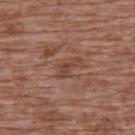This lesion was catalogued during total-body skin photography and was not selected for biopsy. Captured under white-light illumination. A close-up tile cropped from a whole-body skin photograph, about 15 mm across. Located on the back. A female subject aged 73 to 77.